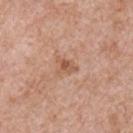notes: imaged on a skin check; not biopsied | location: the chest | patient: male, approximately 65 years of age | acquisition: ~15 mm crop, total-body skin-cancer survey | image-analysis metrics: a mean CIELAB color near L≈56 a*≈22 b*≈32 and a lesion–skin lightness drop of about 9 | illumination: white-light illumination.